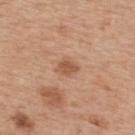Impression: The lesion was tiled from a total-body skin photograph and was not biopsied. Context: Approximately 2.5 mm at its widest. Located on the upper back. The subject is a male in their mid- to late 60s. This is a white-light tile. Cropped from a total-body skin-imaging series; the visible field is about 15 mm. Automated tile analysis of the lesion measured a mean CIELAB color near L≈55 a*≈23 b*≈33, about 10 CIELAB-L* units darker than the surrounding skin, and a normalized border contrast of about 7.